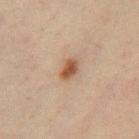Q: What are the patient's age and sex?
A: female, in their mid- to late 40s
Q: Lesion size?
A: about 2.5 mm
Q: What kind of image is this?
A: total-body-photography crop, ~15 mm field of view
Q: Where on the body is the lesion?
A: the right thigh
Q: How was the tile lit?
A: cross-polarized illumination